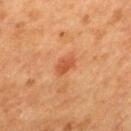Notes:
- size: about 2.5 mm
- image-analysis metrics: a lesion area of about 3.5 mm², a shape eccentricity near 0.8, and a shape-asymmetry score of about 0.25 (0 = symmetric); an average lesion color of about L≈47 a*≈27 b*≈35 (CIELAB), roughly 9 lightness units darker than nearby skin, and a normalized lesion–skin contrast near 6.5; a classifier nevus-likeness of about 25/100 and lesion-presence confidence of about 100/100
- tile lighting: cross-polarized illumination
- anatomic site: the back
- patient: female, about 40 years old
- acquisition: ~15 mm tile from a whole-body skin photo